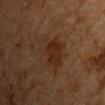notes: no biopsy performed (imaged during a skin exam)
subject: male, approximately 65 years of age
imaging modality: 15 mm crop, total-body photography
automated lesion analysis: a lesion area of about 13 mm² and a shape-asymmetry score of about 0.3 (0 = symmetric); a mean CIELAB color near L≈24 a*≈16 b*≈25, a lesion–skin lightness drop of about 5, and a normalized lesion–skin contrast near 6.5; a color-variation rating of about 3.5/10 and peripheral color asymmetry of about 1
tile lighting: cross-polarized illumination
lesion size: ≈4.5 mm
anatomic site: the front of the torso